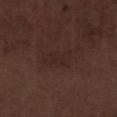workup = catalogued during a skin exam; not biopsied | acquisition = ~15 mm crop, total-body skin-cancer survey | lighting = white-light | anatomic site = the left lower leg | diameter = about 4 mm | patient = male, approximately 70 years of age.